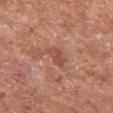Image and clinical context: A male subject, aged 63 to 67. A lesion tile, about 15 mm wide, cut from a 3D total-body photograph. Measured at roughly 3 mm in maximum diameter. The tile uses white-light illumination. From the left thigh.